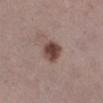biopsy status = no biopsy performed (imaged during a skin exam) | imaging modality = total-body-photography crop, ~15 mm field of view | size = ≈3 mm | site = the right lower leg | patient = female, aged approximately 30.